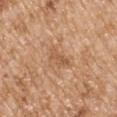Captured during whole-body skin photography for melanoma surveillance; the lesion was not biopsied. About 3 mm across. The lesion is located on the left upper arm. An algorithmic analysis of the crop reported border irregularity of about 5 on a 0–10 scale, a color-variation rating of about 0.5/10, and peripheral color asymmetry of about 0. The software also gave an automated nevus-likeness rating near 0 out of 100 and a lesion-detection confidence of about 100/100. This is a white-light tile. The subject is a male aged approximately 65. This image is a 15 mm lesion crop taken from a total-body photograph.